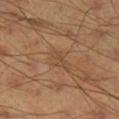Case summary:
* follow-up · imaged on a skin check; not biopsied
* lesion diameter · ~3 mm (longest diameter)
* patient · male, aged approximately 45
* imaging modality · ~15 mm tile from a whole-body skin photo
* automated lesion analysis · a lesion area of about 3 mm² and a shape eccentricity near 0.9; a border-irregularity rating of about 4/10; a classifier nevus-likeness of about 0/100 and lesion-presence confidence of about 95/100
* anatomic site · the right lower leg